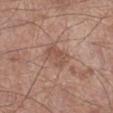  biopsy_status: not biopsied; imaged during a skin examination
  lighting: white-light
  image:
    source: total-body photography crop
    field_of_view_mm: 15
  site: left lower leg
  patient:
    sex: male
    age_approx: 55
  lesion_size:
    long_diameter_mm_approx: 3.0